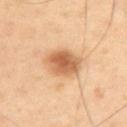Imaged during a routine full-body skin examination; the lesion was not biopsied and no histopathology is available.
Located on the upper back.
Cropped from a total-body skin-imaging series; the visible field is about 15 mm.
A male subject approximately 40 years of age.